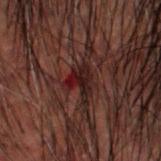Context: The subject is a male in their 60s. Longest diameter approximately 3 mm. Cropped from a whole-body photographic skin survey; the tile spans about 15 mm. Imaged with cross-polarized lighting. From the head or neck.